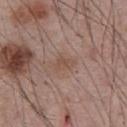notes — total-body-photography surveillance lesion; no biopsy
subject — male, aged 53 to 57
lesion diameter — about 2.5 mm
acquisition — total-body-photography crop, ~15 mm field of view
location — the abdomen